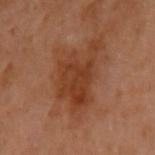workup: catalogued during a skin exam; not biopsied | subject: female, approximately 60 years of age | illumination: cross-polarized | image: ~15 mm crop, total-body skin-cancer survey | site: the arm | lesion size: ≈6.5 mm.